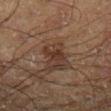Case summary:
* biopsy status · imaged on a skin check; not biopsied